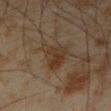notes = catalogued during a skin exam; not biopsied | patient = male, aged around 45 | diameter = ≈5 mm | image = ~15 mm tile from a whole-body skin photo | lighting = cross-polarized | location = the left forearm.